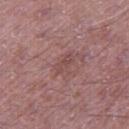Part of a total-body skin-imaging series; this lesion was reviewed on a skin check and was not flagged for biopsy. The total-body-photography lesion software estimated a lesion color around L≈47 a*≈22 b*≈20 in CIELAB and about 6 CIELAB-L* units darker than the surrounding skin. The software also gave a border-irregularity index near 4/10, a color-variation rating of about 0/10, and peripheral color asymmetry of about 0. The patient is a male roughly 50 years of age. A region of skin cropped from a whole-body photographic capture, roughly 15 mm wide. The tile uses white-light illumination. Approximately 2.5 mm at its widest. The lesion is on the right thigh.